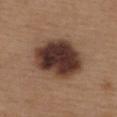workup = catalogued during a skin exam; not biopsied | acquisition = ~15 mm crop, total-body skin-cancer survey | subject = female, aged 38 to 42 | TBP lesion metrics = a lesion area of about 27 mm² and a symmetry-axis asymmetry near 0.1; a lesion color around L≈36 a*≈18 b*≈24 in CIELAB and a normalized border contrast of about 14.5; border irregularity of about 1 on a 0–10 scale, a within-lesion color-variation index near 7.5/10, and a peripheral color-asymmetry measure near 2.5 | location = the upper back | diameter = ~6.5 mm (longest diameter).